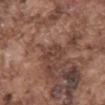biopsy_status: not biopsied; imaged during a skin examination
site: front of the torso
lighting: white-light
patient:
  sex: male
  age_approx: 75
image:
  source: total-body photography crop
  field_of_view_mm: 15
lesion_size:
  long_diameter_mm_approx: 3.0
automated_metrics:
  cielab_L: 40
  cielab_a: 19
  cielab_b: 25
  vs_skin_darker_L: 7.0
  vs_skin_contrast_norm: 6.0